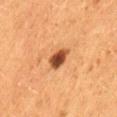biopsy_status: not biopsied; imaged during a skin examination
site: lower back
patient:
  sex: female
  age_approx: 50
image:
  source: total-body photography crop
  field_of_view_mm: 15
lighting: cross-polarized